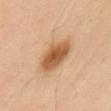Recorded during total-body skin imaging; not selected for excision or biopsy.
This is a cross-polarized tile.
A male subject aged 53 to 57.
Located on the abdomen.
A lesion tile, about 15 mm wide, cut from a 3D total-body photograph.